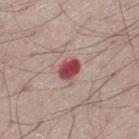Q: Was a biopsy performed?
A: no biopsy performed (imaged during a skin exam)
Q: What did automated image analysis measure?
A: an area of roughly 5 mm², an eccentricity of roughly 0.65, and two-axis asymmetry of about 0.25; a detector confidence of about 100 out of 100 that the crop contains a lesion
Q: What lighting was used for the tile?
A: white-light illumination
Q: What is the lesion's diameter?
A: about 3 mm
Q: Patient demographics?
A: male, approximately 55 years of age
Q: Where on the body is the lesion?
A: the left thigh
Q: How was this image acquired?
A: ~15 mm tile from a whole-body skin photo